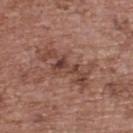Clinical impression: Imaged during a routine full-body skin examination; the lesion was not biopsied and no histopathology is available. Background: Approximately 7 mm at its widest. Cropped from a total-body skin-imaging series; the visible field is about 15 mm. A female subject, aged approximately 65. The lesion-visualizer software estimated an average lesion color of about L≈44 a*≈21 b*≈26 (CIELAB), roughly 9 lightness units darker than nearby skin, and a normalized border contrast of about 7. It also reported a classifier nevus-likeness of about 0/100. The lesion is located on the upper back.